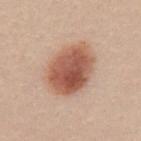Q: Is there a histopathology result?
A: no biopsy performed (imaged during a skin exam)
Q: Where on the body is the lesion?
A: the left upper arm
Q: Patient demographics?
A: female, in their mid- to late 20s
Q: How was this image acquired?
A: 15 mm crop, total-body photography